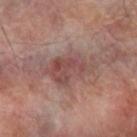biopsy status = imaged on a skin check; not biopsied | image source = 15 mm crop, total-body photography | subject = male, in their 70s | anatomic site = the arm | automated metrics = a footprint of about 15 mm², an eccentricity of roughly 0.85, and a symmetry-axis asymmetry near 0.3; a mean CIELAB color near L≈46 a*≈20 b*≈21; a within-lesion color-variation index near 4.5/10; a classifier nevus-likeness of about 0/100.